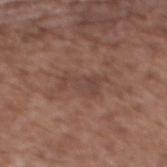follow-up: no biopsy performed (imaged during a skin exam) | diameter: ~5 mm (longest diameter) | tile lighting: white-light illumination | subject: female, in their mid-70s | location: the mid back | image source: 15 mm crop, total-body photography.